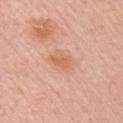<lesion>
<biopsy_status>not biopsied; imaged during a skin examination</biopsy_status>
<patient>
  <sex>female</sex>
  <age_approx>50</age_approx>
</patient>
<lesion_size>
  <long_diameter_mm_approx>2.5</long_diameter_mm_approx>
</lesion_size>
<image>
  <source>total-body photography crop</source>
  <field_of_view_mm>15</field_of_view_mm>
</image>
<site>right upper arm</site>
<automated_metrics>
  <area_mm2_approx>3.0</area_mm2_approx>
  <eccentricity>0.8</eccentricity>
  <shape_asymmetry>0.3</shape_asymmetry>
  <border_irregularity_0_10>3.0</border_irregularity_0_10>
  <color_variation_0_10>1.5</color_variation_0_10>
  <peripheral_color_asymmetry>0.5</peripheral_color_asymmetry>
  <nevus_likeness_0_100>5</nevus_likeness_0_100>
  <lesion_detection_confidence_0_100>100</lesion_detection_confidence_0_100>
</automated_metrics>
<lighting>white-light</lighting>
</lesion>